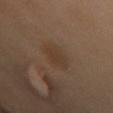biopsy status = total-body-photography surveillance lesion; no biopsy.